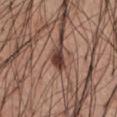follow-up: catalogued during a skin exam; not biopsied
tile lighting: white-light
subject: male, approximately 55 years of age
body site: the abdomen
automated metrics: a lesion area of about 4 mm², an outline eccentricity of about 0.8 (0 = round, 1 = elongated), and a shape-asymmetry score of about 0.3 (0 = symmetric); an average lesion color of about L≈38 a*≈20 b*≈23 (CIELAB), roughly 16 lightness units darker than nearby skin, and a lesion-to-skin contrast of about 12.5 (normalized; higher = more distinct); border irregularity of about 2.5 on a 0–10 scale and a color-variation rating of about 2.5/10
imaging modality: ~15 mm tile from a whole-body skin photo
size: ~2.5 mm (longest diameter)